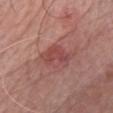<case>
<biopsy_status>not biopsied; imaged during a skin examination</biopsy_status>
<patient>
  <sex>male</sex>
  <age_approx>65</age_approx>
</patient>
<site>chest</site>
<lesion_size>
  <long_diameter_mm_approx>4.5</long_diameter_mm_approx>
</lesion_size>
<image>
  <source>total-body photography crop</source>
  <field_of_view_mm>15</field_of_view_mm>
</image>
<automated_metrics>
  <color_variation_0_10>4.5</color_variation_0_10>
  <peripheral_color_asymmetry>1.5</peripheral_color_asymmetry>
  <nevus_likeness_0_100>30</nevus_likeness_0_100>
  <lesion_detection_confidence_0_100>100</lesion_detection_confidence_0_100>
</automated_metrics>
</case>